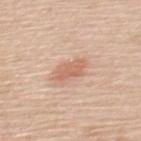Notes:
* biopsy status: imaged on a skin check; not biopsied